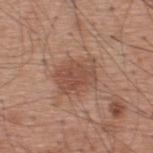The lesion was tiled from a total-body skin photograph and was not biopsied.
An algorithmic analysis of the crop reported an outline eccentricity of about 0.65 (0 = round, 1 = elongated) and a symmetry-axis asymmetry near 0.15. The analysis additionally found a lesion color around L≈50 a*≈21 b*≈28 in CIELAB, roughly 8 lightness units darker than nearby skin, and a lesion-to-skin contrast of about 6.5 (normalized; higher = more distinct). The analysis additionally found border irregularity of about 2 on a 0–10 scale and internal color variation of about 4 on a 0–10 scale. The software also gave an automated nevus-likeness rating near 90 out of 100 and lesion-presence confidence of about 100/100.
The lesion's longest dimension is about 4.5 mm.
A male patient aged approximately 60.
From the upper back.
A region of skin cropped from a whole-body photographic capture, roughly 15 mm wide.